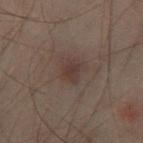The lesion was tiled from a total-body skin photograph and was not biopsied.
A 15 mm close-up extracted from a 3D total-body photography capture.
Automated image analysis of the tile measured an area of roughly 4 mm², an eccentricity of roughly 0.7, and a symmetry-axis asymmetry near 0.3. It also reported border irregularity of about 3 on a 0–10 scale, a within-lesion color-variation index near 2.5/10, and peripheral color asymmetry of about 1. And it measured a nevus-likeness score of about 5/100 and a lesion-detection confidence of about 100/100.
About 2.5 mm across.
A male patient, about 50 years old.
This is a cross-polarized tile.
The lesion is on the left leg.